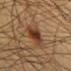Imaged during a routine full-body skin examination; the lesion was not biopsied and no histopathology is available.
This is a cross-polarized tile.
Cropped from a total-body skin-imaging series; the visible field is about 15 mm.
On the chest.
The lesion-visualizer software estimated a footprint of about 10 mm², an eccentricity of roughly 0.8, and a symmetry-axis asymmetry near 0.25. It also reported a classifier nevus-likeness of about 90/100 and a detector confidence of about 100 out of 100 that the crop contains a lesion.
The subject is a male aged around 60.
Measured at roughly 5 mm in maximum diameter.